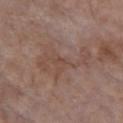workup — total-body-photography surveillance lesion; no biopsy
patient — female, about 85 years old or older
image-analysis metrics — a lesion color around L≈47 a*≈17 b*≈24 in CIELAB, a lesion–skin lightness drop of about 6, and a normalized lesion–skin contrast near 5; a border-irregularity index near 9/10, internal color variation of about 2 on a 0–10 scale, and radial color variation of about 0.5; a classifier nevus-likeness of about 0/100
acquisition — ~15 mm crop, total-body skin-cancer survey
illumination — white-light
anatomic site — the chest